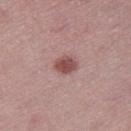About 2.5 mm across.
The lesion is on the right thigh.
A female subject approximately 45 years of age.
A region of skin cropped from a whole-body photographic capture, roughly 15 mm wide.